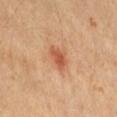| field | value |
|---|---|
| follow-up | catalogued during a skin exam; not biopsied |
| size | ≈3 mm |
| body site | the abdomen |
| subject | female, in their 40s |
| illumination | cross-polarized |
| image source | ~15 mm tile from a whole-body skin photo |
| automated metrics | an area of roughly 4.5 mm², a shape eccentricity near 0.75, and a shape-asymmetry score of about 0.4 (0 = symmetric); a lesion–skin lightness drop of about 11; border irregularity of about 3.5 on a 0–10 scale, a within-lesion color-variation index near 3/10, and radial color variation of about 1; a nevus-likeness score of about 85/100 |